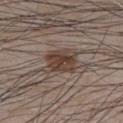The lesion was tiled from a total-body skin photograph and was not biopsied.
The lesion is located on the abdomen.
This image is a 15 mm lesion crop taken from a total-body photograph.
A male patient aged approximately 80.
Captured under white-light illumination.